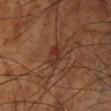{
  "biopsy_status": "not biopsied; imaged during a skin examination",
  "image": {
    "source": "total-body photography crop",
    "field_of_view_mm": 15
  },
  "lesion_size": {
    "long_diameter_mm_approx": 3.0
  },
  "patient": {
    "sex": "male",
    "age_approx": 70
  },
  "lighting": "cross-polarized",
  "site": "left lower leg"
}